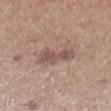workup = catalogued during a skin exam; not biopsied
site = the left upper arm
patient = female, about 65 years old
image = ~15 mm tile from a whole-body skin photo